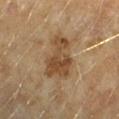No biopsy was performed on this lesion — it was imaged during a full skin examination and was not determined to be concerning.
This is a cross-polarized tile.
A 15 mm close-up tile from a total-body photography series done for melanoma screening.
Longest diameter approximately 6 mm.
The lesion is located on the left forearm.
A female subject aged approximately 60.